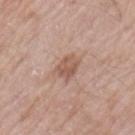Q: Was a biopsy performed?
A: no biopsy performed (imaged during a skin exam)
Q: How was the tile lit?
A: white-light illumination
Q: Who is the patient?
A: male, in their 60s
Q: Lesion size?
A: ≈3.5 mm
Q: What is the imaging modality?
A: 15 mm crop, total-body photography
Q: What is the anatomic site?
A: the left upper arm